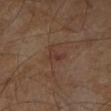Background:
The recorded lesion diameter is about 3 mm. The lesion-visualizer software estimated internal color variation of about 3.5 on a 0–10 scale and radial color variation of about 1. A male patient, roughly 60 years of age. Imaged with cross-polarized lighting. A close-up tile cropped from a whole-body skin photograph, about 15 mm across. The lesion is located on the right leg.